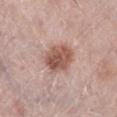| feature | finding |
|---|---|
| notes | no biopsy performed (imaged during a skin exam) |
| site | the right lower leg |
| subject | female, aged approximately 65 |
| illumination | white-light illumination |
| diameter | about 4.5 mm |
| acquisition | ~15 mm crop, total-body skin-cancer survey |